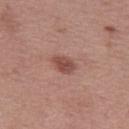biopsy status: imaged on a skin check; not biopsied
image: ~15 mm tile from a whole-body skin photo
tile lighting: white-light
anatomic site: the left thigh
patient: female, about 50 years old
diameter: about 3.5 mm